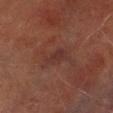Clinical summary: Located on the right lower leg. A lesion tile, about 15 mm wide, cut from a 3D total-body photograph. This is a cross-polarized tile. A male patient roughly 70 years of age. The lesion's longest dimension is about 3.5 mm.